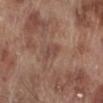- biopsy status · no biopsy performed (imaged during a skin exam)
- anatomic site · the right lower leg
- subject · male, aged 68 to 72
- tile lighting · white-light
- acquisition · ~15 mm tile from a whole-body skin photo